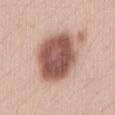Case summary:
• follow-up: total-body-photography surveillance lesion; no biopsy
• anatomic site: the abdomen
• patient: female, aged 18–22
• TBP lesion metrics: a border-irregularity rating of about 1/10 and internal color variation of about 5.5 on a 0–10 scale; a classifier nevus-likeness of about 90/100
• lesion size: ≈7 mm
• image: 15 mm crop, total-body photography